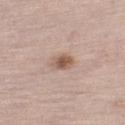Clinical impression: Captured during whole-body skin photography for melanoma surveillance; the lesion was not biopsied. Background: The lesion is located on the left lower leg. Cropped from a total-body skin-imaging series; the visible field is about 15 mm. A male subject approximately 80 years of age. The tile uses white-light illumination. Approximately 2.5 mm at its widest.